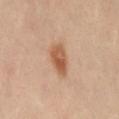biopsy status=imaged on a skin check; not biopsied | illumination=cross-polarized illumination | location=the lower back | imaging modality=15 mm crop, total-body photography | subject=female, aged approximately 40.